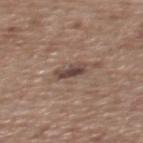Imaged during a routine full-body skin examination; the lesion was not biopsied and no histopathology is available. A close-up tile cropped from a whole-body skin photograph, about 15 mm across. Located on the mid back. About 3.5 mm across. A male patient, aged 63 to 67.